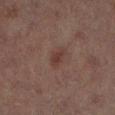Clinical impression:
The lesion was tiled from a total-body skin photograph and was not biopsied.
Clinical summary:
The lesion is located on the left lower leg. About 2.5 mm across. This image is a 15 mm lesion crop taken from a total-body photograph. A female patient aged around 70. The tile uses cross-polarized illumination.